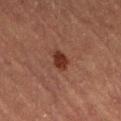| feature | finding |
|---|---|
| biopsy status | total-body-photography surveillance lesion; no biopsy |
| size | ~2.5 mm (longest diameter) |
| patient | female, approximately 70 years of age |
| site | the leg |
| automated lesion analysis | a detector confidence of about 100 out of 100 that the crop contains a lesion |
| image | ~15 mm tile from a whole-body skin photo |
| lighting | cross-polarized illumination |